A 15 mm close-up tile from a total-body photography series done for melanoma screening · the lesion is located on the upper back · a male subject, in their mid-60s: 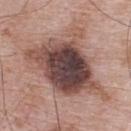lesion diameter=about 11.5 mm | illumination=white-light | biopsy diagnosis=a dysplastic (Clark) nevus.